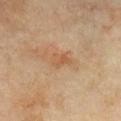{
  "patient": {
    "sex": "female",
    "age_approx": 70
  },
  "site": "chest",
  "lighting": "cross-polarized",
  "image": {
    "source": "total-body photography crop",
    "field_of_view_mm": 15
  },
  "automated_metrics": {
    "area_mm2_approx": 4.0,
    "eccentricity": 0.75,
    "shape_asymmetry": 0.25,
    "border_irregularity_0_10": 2.5,
    "color_variation_0_10": 2.5,
    "peripheral_color_asymmetry": 1.0
  }
}